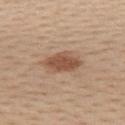Part of a total-body skin-imaging series; this lesion was reviewed on a skin check and was not flagged for biopsy. Captured under white-light illumination. A female patient, aged around 40. A roughly 15 mm field-of-view crop from a total-body skin photograph. From the back.